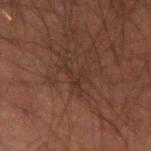Recorded during total-body skin imaging; not selected for excision or biopsy. A lesion tile, about 15 mm wide, cut from a 3D total-body photograph. The recorded lesion diameter is about 4 mm. Captured under cross-polarized illumination. A male subject about 50 years old. On the left forearm.